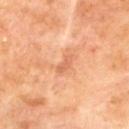Recorded during total-body skin imaging; not selected for excision or biopsy. The lesion is on the mid back. A male subject in their 70s. This is a cross-polarized tile. An algorithmic analysis of the crop reported an area of roughly 2.5 mm² and two-axis asymmetry of about 0.4. It also reported a border-irregularity rating of about 4/10 and a peripheral color-asymmetry measure near 0. The software also gave an automated nevus-likeness rating near 0 out of 100 and a lesion-detection confidence of about 100/100. A close-up tile cropped from a whole-body skin photograph, about 15 mm across.